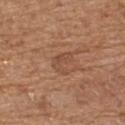The lesion is on the upper back.
This is a white-light tile.
The total-body-photography lesion software estimated an eccentricity of roughly 0.6 and two-axis asymmetry of about 0.55. And it measured an average lesion color of about L≈49 a*≈21 b*≈31 (CIELAB) and a lesion-to-skin contrast of about 5 (normalized; higher = more distinct). The software also gave a nevus-likeness score of about 0/100 and lesion-presence confidence of about 100/100.
A male subject aged around 70.
A roughly 15 mm field-of-view crop from a total-body skin photograph.
Approximately 2.5 mm at its widest.